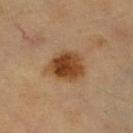  site: leg
  patient:
    sex: female
    age_approx: 70
  automated_metrics:
    area_mm2_approx: 12.0
    shape_asymmetry: 0.2
    border_irregularity_0_10: 2.0
    peripheral_color_asymmetry: 2.0
  image:
    source: total-body photography crop
    field_of_view_mm: 15
  lighting: cross-polarized
  lesion_size:
    long_diameter_mm_approx: 4.5